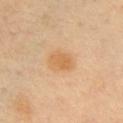Captured during whole-body skin photography for melanoma surveillance; the lesion was not biopsied. The lesion's longest dimension is about 3 mm. The total-body-photography lesion software estimated a footprint of about 6.5 mm², an outline eccentricity of about 0.7 (0 = round, 1 = elongated), and a symmetry-axis asymmetry near 0.2. It also reported a mean CIELAB color near L≈64 a*≈20 b*≈42, a lesion–skin lightness drop of about 8, and a normalized lesion–skin contrast near 6.5. And it measured a border-irregularity rating of about 1.5/10 and a within-lesion color-variation index near 2.5/10. It also reported an automated nevus-likeness rating near 70 out of 100 and a detector confidence of about 100 out of 100 that the crop contains a lesion. Imaged with cross-polarized lighting. From the chest. A lesion tile, about 15 mm wide, cut from a 3D total-body photograph. A male patient, about 40 years old.